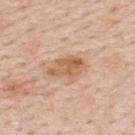{
  "biopsy_status": "not biopsied; imaged during a skin examination",
  "site": "mid back",
  "patient": {
    "sex": "male",
    "age_approx": 60
  },
  "image": {
    "source": "total-body photography crop",
    "field_of_view_mm": 15
  }
}